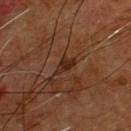| key | value |
|---|---|
| workup | catalogued during a skin exam; not biopsied |
| image source | 15 mm crop, total-body photography |
| automated lesion analysis | a lesion area of about 3 mm² and a shape-asymmetry score of about 0.35 (0 = symmetric); a lesion color around L≈24 a*≈20 b*≈26 in CIELAB and roughly 7 lightness units darker than nearby skin |
| site | the front of the torso |
| diameter | ≈3 mm |
| patient | male, approximately 55 years of age |